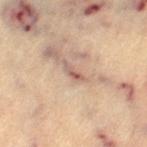No biopsy was performed on this lesion — it was imaged during a full skin examination and was not determined to be concerning.
The lesion-visualizer software estimated an average lesion color of about L≈55 a*≈20 b*≈25 (CIELAB), roughly 11 lightness units darker than nearby skin, and a normalized border contrast of about 7.5. The analysis additionally found a color-variation rating of about 0/10.
A female patient, about 65 years old.
Located on the left thigh.
About 2.5 mm across.
A close-up tile cropped from a whole-body skin photograph, about 15 mm across.
Imaged with cross-polarized lighting.